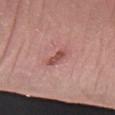| key | value |
|---|---|
| notes | imaged on a skin check; not biopsied |
| site | the right forearm |
| acquisition | ~15 mm crop, total-body skin-cancer survey |
| automated lesion analysis | a lesion area of about 4 mm², an eccentricity of roughly 0.9, and a symmetry-axis asymmetry near 0.25; internal color variation of about 2 on a 0–10 scale and a peripheral color-asymmetry measure near 0.5; a nevus-likeness score of about 15/100 |
| subject | male, in their mid-70s |
| illumination | white-light illumination |